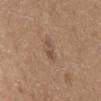Q: Was a biopsy performed?
A: no biopsy performed (imaged during a skin exam)
Q: What kind of image is this?
A: ~15 mm tile from a whole-body skin photo
Q: Where on the body is the lesion?
A: the mid back
Q: Lesion size?
A: ≈2.5 mm
Q: What did automated image analysis measure?
A: a lesion area of about 3.5 mm², a shape eccentricity near 0.8, and a shape-asymmetry score of about 0.3 (0 = symmetric); a mean CIELAB color near L≈50 a*≈17 b*≈28, roughly 7 lightness units darker than nearby skin, and a normalized border contrast of about 5.5; a border-irregularity rating of about 3.5/10, a color-variation rating of about 2.5/10, and radial color variation of about 0.5
Q: What are the patient's age and sex?
A: male, aged around 65